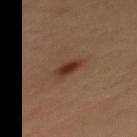Findings:
– follow-up: total-body-photography surveillance lesion; no biopsy
– image source: total-body-photography crop, ~15 mm field of view
– patient: male, aged approximately 65
– anatomic site: the mid back